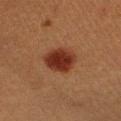Findings:
- follow-up: imaged on a skin check; not biopsied
- tile lighting: cross-polarized
- lesion diameter: ~4.5 mm (longest diameter)
- acquisition: ~15 mm crop, total-body skin-cancer survey
- patient: male, aged around 40
- anatomic site: the left upper arm